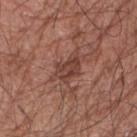Clinical impression: Recorded during total-body skin imaging; not selected for excision or biopsy. Acquisition and patient details: Cropped from a whole-body photographic skin survey; the tile spans about 15 mm. An algorithmic analysis of the crop reported a lesion area of about 8.5 mm² and a shape-asymmetry score of about 0.35 (0 = symmetric). And it measured a lesion color around L≈42 a*≈22 b*≈25 in CIELAB, roughly 9 lightness units darker than nearby skin, and a normalized border contrast of about 7. The subject is a male about 65 years old. From the right upper arm. Imaged with white-light lighting. Measured at roughly 5 mm in maximum diameter.